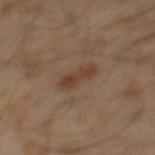Recorded during total-body skin imaging; not selected for excision or biopsy. A male patient roughly 60 years of age. The lesion is on the abdomen. Longest diameter approximately 4 mm. Cropped from a whole-body photographic skin survey; the tile spans about 15 mm. The total-body-photography lesion software estimated a symmetry-axis asymmetry near 0.15.